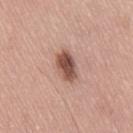biopsy status = total-body-photography surveillance lesion; no biopsy | acquisition = ~15 mm tile from a whole-body skin photo | anatomic site = the right thigh | subject = male, in their mid-50s.